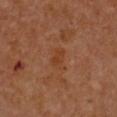The lesion was tiled from a total-body skin photograph and was not biopsied. About 2.5 mm across. Automated image analysis of the tile measured an outline eccentricity of about 0.8 (0 = round, 1 = elongated) and a shape-asymmetry score of about 0.3 (0 = symmetric). The software also gave a border-irregularity rating of about 3/10 and a peripheral color-asymmetry measure near 0.5. And it measured an automated nevus-likeness rating near 0 out of 100. A close-up tile cropped from a whole-body skin photograph, about 15 mm across. Imaged with cross-polarized lighting. Located on the chest. A female subject, aged approximately 55.